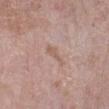Recorded during total-body skin imaging; not selected for excision or biopsy. This image is a 15 mm lesion crop taken from a total-body photograph. A female patient aged 68–72. Located on the left lower leg. This is a white-light tile. About 3 mm across.